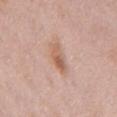Q: What is the imaging modality?
A: ~15 mm crop, total-body skin-cancer survey
Q: Lesion size?
A: ≈3.5 mm
Q: What lighting was used for the tile?
A: white-light illumination
Q: Patient demographics?
A: male, aged 73 to 77
Q: Where on the body is the lesion?
A: the mid back